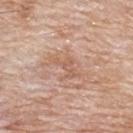Recorded during total-body skin imaging; not selected for excision or biopsy.
A male subject, in their 80s.
A lesion tile, about 15 mm wide, cut from a 3D total-body photograph.
Located on the upper back.
Measured at roughly 3 mm in maximum diameter.